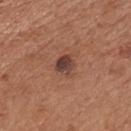biopsy status: no biopsy performed (imaged during a skin exam) | patient: male, about 55 years old | image: ~15 mm crop, total-body skin-cancer survey | site: the chest | lesion size: ~2.5 mm (longest diameter).